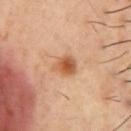No biopsy was performed on this lesion — it was imaged during a full skin examination and was not determined to be concerning. The tile uses cross-polarized illumination. The subject is a male about 55 years old. A 15 mm close-up tile from a total-body photography series done for melanoma screening. The recorded lesion diameter is about 2.5 mm. The lesion-visualizer software estimated a footprint of about 5.5 mm², a shape eccentricity near 0.5, and a symmetry-axis asymmetry near 0.25. It also reported a border-irregularity rating of about 2/10, internal color variation of about 5 on a 0–10 scale, and a peripheral color-asymmetry measure near 1.5. From the chest.